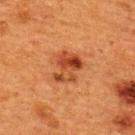biopsy status: total-body-photography surveillance lesion; no biopsy
lesion size: ≈3.5 mm
image source: ~15 mm crop, total-body skin-cancer survey
subject: female, aged approximately 40
body site: the upper back
automated metrics: a lesion area of about 8.5 mm², an outline eccentricity of about 0.65 (0 = round, 1 = elongated), and two-axis asymmetry of about 0.3; border irregularity of about 4 on a 0–10 scale, a color-variation rating of about 9/10, and a peripheral color-asymmetry measure near 3; an automated nevus-likeness rating near 80 out of 100 and a lesion-detection confidence of about 100/100